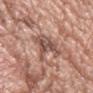Assessment:
Recorded during total-body skin imaging; not selected for excision or biopsy.
Context:
Automated tile analysis of the lesion measured an area of roughly 10 mm², an outline eccentricity of about 0.8 (0 = round, 1 = elongated), and a shape-asymmetry score of about 0.4 (0 = symmetric). A 15 mm crop from a total-body photograph taken for skin-cancer surveillance. The lesion is on the chest. Imaged with white-light lighting. A male patient, roughly 60 years of age. The recorded lesion diameter is about 5.5 mm.